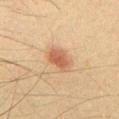  biopsy_status: not biopsied; imaged during a skin examination
  image:
    source: total-body photography crop
    field_of_view_mm: 15
  site: chest
  lighting: cross-polarized
  automated_metrics:
    area_mm2_approx: 6.0
    eccentricity: 0.75
    shape_asymmetry: 0.25
    border_irregularity_0_10: 2.5
    color_variation_0_10: 2.5
    peripheral_color_asymmetry: 1.0
    nevus_likeness_0_100: 100
  lesion_size:
    long_diameter_mm_approx: 3.5
  patient:
    sex: male
    age_approx: 35